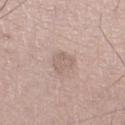Impression:
The lesion was tiled from a total-body skin photograph and was not biopsied.
Image and clinical context:
Imaged with white-light lighting. The lesion is located on the left lower leg. A roughly 15 mm field-of-view crop from a total-body skin photograph. The patient is a male in their 60s.